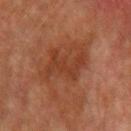The lesion was tiled from a total-body skin photograph and was not biopsied.
A male subject, aged 73 to 77.
This is a cross-polarized tile.
A lesion tile, about 15 mm wide, cut from a 3D total-body photograph.
Approximately 5 mm at its widest.
From the left upper arm.